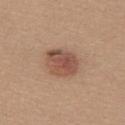Q: Was a biopsy performed?
A: catalogued during a skin exam; not biopsied
Q: What is the imaging modality?
A: ~15 mm crop, total-body skin-cancer survey
Q: Who is the patient?
A: female, aged approximately 20
Q: What is the anatomic site?
A: the upper back
Q: What lighting was used for the tile?
A: white-light illumination